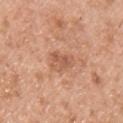The lesion was photographed on a routine skin check and not biopsied; there is no pathology result. From the chest. Cropped from a whole-body photographic skin survey; the tile spans about 15 mm. Imaged with white-light lighting. A male subject aged 28–32. The recorded lesion diameter is about 3 mm.